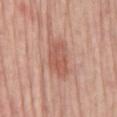workup = total-body-photography surveillance lesion; no biopsy | size = ~5 mm (longest diameter) | subject = female, aged 68–72 | image source = 15 mm crop, total-body photography | location = the mid back | image-analysis metrics = a footprint of about 11 mm², an eccentricity of roughly 0.85, and two-axis asymmetry of about 0.25; a color-variation rating of about 3/10 and radial color variation of about 1; a detector confidence of about 100 out of 100 that the crop contains a lesion | tile lighting = white-light.